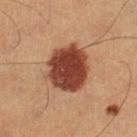Notes:
• lesion size: about 5.5 mm
• subject: male, roughly 60 years of age
• body site: the left lower leg
• image: ~15 mm tile from a whole-body skin photo
• illumination: cross-polarized illumination
• image-analysis metrics: a lesion area of about 21 mm², a shape eccentricity near 0.55, and two-axis asymmetry of about 0.1; a lesion color around L≈34 a*≈22 b*≈26 in CIELAB, roughly 16 lightness units darker than nearby skin, and a normalized lesion–skin contrast near 13.5; a border-irregularity rating of about 1.5/10, a color-variation rating of about 4/10, and a peripheral color-asymmetry measure near 1.5